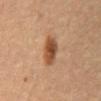Part of a total-body skin-imaging series; this lesion was reviewed on a skin check and was not flagged for biopsy.